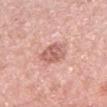Q: Was a biopsy performed?
A: no biopsy performed (imaged during a skin exam)
Q: How was the tile lit?
A: white-light
Q: Automated lesion metrics?
A: a mean CIELAB color near L≈61 a*≈25 b*≈26, a lesion–skin lightness drop of about 11, and a normalized lesion–skin contrast near 7; a border-irregularity index near 2/10, internal color variation of about 3.5 on a 0–10 scale, and a peripheral color-asymmetry measure near 1.5
Q: What is the anatomic site?
A: the left forearm
Q: What is the lesion's diameter?
A: about 3 mm
Q: What are the patient's age and sex?
A: female, aged 38–42
Q: What is the imaging modality?
A: 15 mm crop, total-body photography